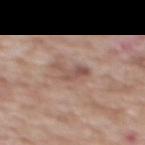Assessment: The lesion was photographed on a routine skin check and not biopsied; there is no pathology result. Background: This image is a 15 mm lesion crop taken from a total-body photograph. The lesion is located on the chest. Captured under white-light illumination. Approximately 3.5 mm at its widest. Automated tile analysis of the lesion measured a lesion area of about 4 mm², a shape eccentricity near 0.8, and a symmetry-axis asymmetry near 0.5. A male patient aged 58–62.